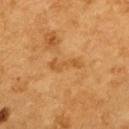Q: Was a biopsy performed?
A: no biopsy performed (imaged during a skin exam)
Q: How was the tile lit?
A: cross-polarized
Q: Where on the body is the lesion?
A: the back
Q: What kind of image is this?
A: total-body-photography crop, ~15 mm field of view
Q: What did automated image analysis measure?
A: an area of roughly 4 mm² and two-axis asymmetry of about 0.45; roughly 8 lightness units darker than nearby skin and a normalized lesion–skin contrast near 5.5
Q: Patient demographics?
A: female, aged around 55
Q: How large is the lesion?
A: ~4 mm (longest diameter)